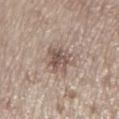The lesion was tiled from a total-body skin photograph and was not biopsied. A region of skin cropped from a whole-body photographic capture, roughly 15 mm wide. Located on the left lower leg. A male subject, aged approximately 70.